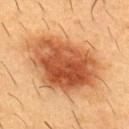notes — total-body-photography surveillance lesion; no biopsy
patient — male, in their mid-50s
anatomic site — the chest
illumination — cross-polarized
lesion diameter — ≈9.5 mm
imaging modality — ~15 mm tile from a whole-body skin photo